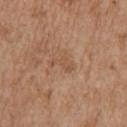Findings:
- subject · female, aged around 75
- diameter · ≈3 mm
- illumination · white-light
- acquisition · total-body-photography crop, ~15 mm field of view
- location · the right upper arm